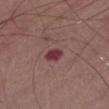Clinical impression: Captured during whole-body skin photography for melanoma surveillance; the lesion was not biopsied. Acquisition and patient details: Imaged with white-light lighting. Automated tile analysis of the lesion measured a footprint of about 3.5 mm², an eccentricity of roughly 0.8, and two-axis asymmetry of about 0.2. The analysis additionally found a border-irregularity rating of about 1.5/10, a color-variation rating of about 2/10, and peripheral color asymmetry of about 0.5. And it measured a lesion-detection confidence of about 100/100. A close-up tile cropped from a whole-body skin photograph, about 15 mm across. The lesion is on the abdomen. A male subject aged approximately 75. Approximately 2.5 mm at its widest.